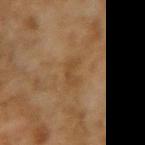| key | value |
|---|---|
| workup | no biopsy performed (imaged during a skin exam) |
| image source | total-body-photography crop, ~15 mm field of view |
| subject | female, aged approximately 60 |
| body site | the left upper arm |